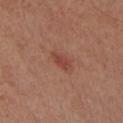Part of a total-body skin-imaging series; this lesion was reviewed on a skin check and was not flagged for biopsy. On the chest. The subject is a male aged around 55. Captured under white-light illumination. The total-body-photography lesion software estimated an area of roughly 3 mm² and a shape eccentricity near 0.9. The software also gave a lesion–skin lightness drop of about 8 and a normalized lesion–skin contrast near 6.5. A close-up tile cropped from a whole-body skin photograph, about 15 mm across. The lesion's longest dimension is about 2.5 mm.